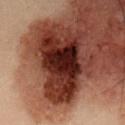follow-up — catalogued during a skin exam; not biopsied